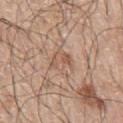Impression: This lesion was catalogued during total-body skin photography and was not selected for biopsy. Image and clinical context: Imaged with white-light lighting. Automated tile analysis of the lesion measured a footprint of about 5.5 mm², a shape eccentricity near 0.55, and a shape-asymmetry score of about 0.3 (0 = symmetric). And it measured a border-irregularity rating of about 3.5/10. Measured at roughly 3 mm in maximum diameter. From the left arm. The patient is a male aged 68–72. A 15 mm crop from a total-body photograph taken for skin-cancer surveillance.